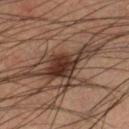Q: Was this lesion biopsied?
A: catalogued during a skin exam; not biopsied
Q: What are the patient's age and sex?
A: male, aged 63–67
Q: Lesion location?
A: the right lower leg
Q: How was the tile lit?
A: cross-polarized illumination
Q: What kind of image is this?
A: ~15 mm crop, total-body skin-cancer survey
Q: Lesion size?
A: ~5.5 mm (longest diameter)